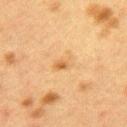Assessment: The lesion was photographed on a routine skin check and not biopsied; there is no pathology result. Background: This is a cross-polarized tile. Approximately 3 mm at its widest. The lesion is on the mid back. A female patient roughly 40 years of age. Automated image analysis of the tile measured a border-irregularity rating of about 3/10, a color-variation rating of about 4.5/10, and peripheral color asymmetry of about 1.5. This image is a 15 mm lesion crop taken from a total-body photograph.